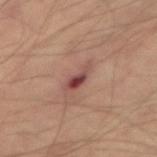  biopsy_status: not biopsied; imaged during a skin examination
  lighting: cross-polarized
  site: abdomen
  patient:
    sex: male
    age_approx: 65
  lesion_size:
    long_diameter_mm_approx: 3.5
  image:
    source: total-body photography crop
    field_of_view_mm: 15